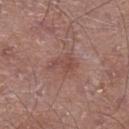Captured during whole-body skin photography for melanoma surveillance; the lesion was not biopsied.
The lesion is on the leg.
Captured under white-light illumination.
The lesion's longest dimension is about 3.5 mm.
A roughly 15 mm field-of-view crop from a total-body skin photograph.
The patient is a male aged around 70.